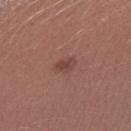The lesion is on the left forearm.
A 15 mm crop from a total-body photograph taken for skin-cancer surveillance.
Longest diameter approximately 2.5 mm.
Automated tile analysis of the lesion measured a nevus-likeness score of about 60/100 and lesion-presence confidence of about 100/100.
A female subject, approximately 25 years of age.